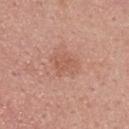biopsy_status: not biopsied; imaged during a skin examination
lighting: white-light
automated_metrics:
  area_mm2_approx: 6.0
  eccentricity: 0.6
  border_irregularity_0_10: 3.5
  color_variation_0_10: 1.5
  peripheral_color_asymmetry: 0.5
  nevus_likeness_0_100: 0
  lesion_detection_confidence_0_100: 100
image:
  source: total-body photography crop
  field_of_view_mm: 15
patient:
  sex: male
  age_approx: 25
site: upper back